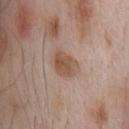notes — imaged on a skin check; not biopsied
image — 15 mm crop, total-body photography
anatomic site — the chest
tile lighting — white-light
patient — male, aged 58 to 62